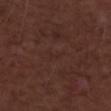A 15 mm close-up extracted from a 3D total-body photography capture. A female patient aged around 50. The lesion is on the arm. Histopathological examination showed a malignancy: nodular basal cell carcinoma.